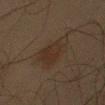  biopsy_status: not biopsied; imaged during a skin examination
  image:
    source: total-body photography crop
    field_of_view_mm: 15
  lighting: cross-polarized
  automated_metrics:
    shape_asymmetry: 0.35
    cielab_L: 23
    cielab_a: 11
    cielab_b: 20
    border_irregularity_0_10: 3.5
    color_variation_0_10: 2.5
    peripheral_color_asymmetry: 1.0
  patient:
    sex: male
    age_approx: 45
  site: left upper arm